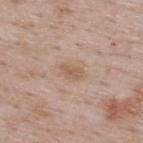Notes:
- image — total-body-photography crop, ~15 mm field of view
- anatomic site — the back
- patient — male, approximately 50 years of age